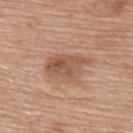Impression:
Captured during whole-body skin photography for melanoma surveillance; the lesion was not biopsied.
Context:
A region of skin cropped from a whole-body photographic capture, roughly 15 mm wide. Longest diameter approximately 5 mm. An algorithmic analysis of the crop reported a border-irregularity index near 3.5/10, a color-variation rating of about 5/10, and radial color variation of about 2. The software also gave a classifier nevus-likeness of about 15/100 and lesion-presence confidence of about 100/100. The lesion is located on the upper back. A female subject, aged approximately 60. Imaged with white-light lighting.